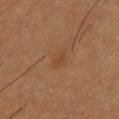<tbp_lesion>
<biopsy_status>not biopsied; imaged during a skin examination</biopsy_status>
<site>upper back</site>
<lesion_size>
  <long_diameter_mm_approx>2.5</long_diameter_mm_approx>
</lesion_size>
<image>
  <source>total-body photography crop</source>
  <field_of_view_mm>15</field_of_view_mm>
</image>
<lighting>cross-polarized</lighting>
<patient>
  <sex>male</sex>
  <age_approx>55</age_approx>
</patient>
</tbp_lesion>